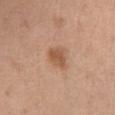notes: total-body-photography surveillance lesion; no biopsy | acquisition: total-body-photography crop, ~15 mm field of view | patient: female, aged 48–52 | automated lesion analysis: an area of roughly 5.5 mm², a shape eccentricity near 0.7, and a symmetry-axis asymmetry near 0.3; an average lesion color of about L≈55 a*≈22 b*≈33 (CIELAB), a lesion–skin lightness drop of about 10, and a normalized border contrast of about 7; an automated nevus-likeness rating near 90 out of 100 | body site: the chest | tile lighting: white-light illumination.